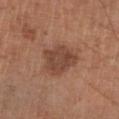Acquisition and patient details: The lesion is located on the left lower leg. A male patient, aged approximately 65. A roughly 15 mm field-of-view crop from a total-body skin photograph. Automated tile analysis of the lesion measured an average lesion color of about L≈41 a*≈19 b*≈27 (CIELAB), about 9 CIELAB-L* units darker than the surrounding skin, and a lesion-to-skin contrast of about 7.5 (normalized; higher = more distinct). And it measured a classifier nevus-likeness of about 20/100 and a detector confidence of about 100 out of 100 that the crop contains a lesion.